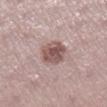Recorded during total-body skin imaging; not selected for excision or biopsy.